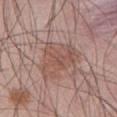notes: catalogued during a skin exam; not biopsied
site: the front of the torso
image source: ~15 mm tile from a whole-body skin photo
patient: male, approximately 55 years of age
illumination: white-light illumination